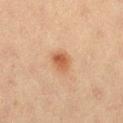Clinical impression:
The lesion was photographed on a routine skin check and not biopsied; there is no pathology result.
Context:
The patient is a female roughly 20 years of age. Captured under cross-polarized illumination. On the left thigh. Longest diameter approximately 2.5 mm. A 15 mm crop from a total-body photograph taken for skin-cancer surveillance.